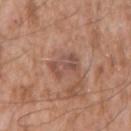Q: What are the patient's age and sex?
A: male, aged around 60
Q: What is the imaging modality?
A: 15 mm crop, total-body photography
Q: What lighting was used for the tile?
A: white-light
Q: What is the anatomic site?
A: the left upper arm